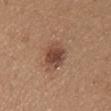Impression:
The lesion was tiled from a total-body skin photograph and was not biopsied.
Context:
The subject is a female aged 43–47. Imaged with white-light lighting. From the right upper arm. A 15 mm close-up extracted from a 3D total-body photography capture. The lesion's longest dimension is about 3.5 mm.